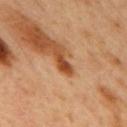Clinical impression: The lesion was tiled from a total-body skin photograph and was not biopsied. Context: From the mid back. The lesion's longest dimension is about 2.5 mm. The patient is a male aged approximately 50. A 15 mm close-up extracted from a 3D total-body photography capture.